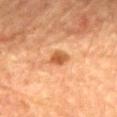| key | value |
|---|---|
| notes | catalogued during a skin exam; not biopsied |
| image | 15 mm crop, total-body photography |
| patient | female, aged 68 to 72 |
| TBP lesion metrics | an average lesion color of about L≈48 a*≈24 b*≈37 (CIELAB), roughly 11 lightness units darker than nearby skin, and a normalized border contrast of about 8.5; a border-irregularity rating of about 2.5/10 and peripheral color asymmetry of about 0.5 |
| site | the back |
| size | ≈2.5 mm |
| lighting | cross-polarized |